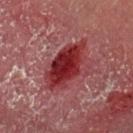Cropped from a total-body skin-imaging series; the visible field is about 15 mm. Located on the left upper arm. The patient is a male aged 53–57.